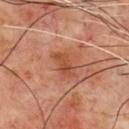notes = total-body-photography surveillance lesion; no biopsy.